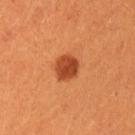This lesion was catalogued during total-body skin photography and was not selected for biopsy.
From the left upper arm.
A lesion tile, about 15 mm wide, cut from a 3D total-body photograph.
A female subject, approximately 30 years of age.